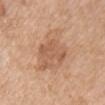biopsy status: catalogued during a skin exam; not biopsied
location: the chest
image: 15 mm crop, total-body photography
subject: female, in their 40s